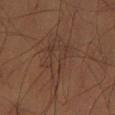This lesion was catalogued during total-body skin photography and was not selected for biopsy. A lesion tile, about 15 mm wide, cut from a 3D total-body photograph. The lesion is located on the left thigh. Captured under cross-polarized illumination. A male subject, aged 58–62.